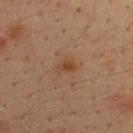No biopsy was performed on this lesion — it was imaged during a full skin examination and was not determined to be concerning. A region of skin cropped from a whole-body photographic capture, roughly 15 mm wide. The tile uses cross-polarized illumination. Automated image analysis of the tile measured about 6 CIELAB-L* units darker than the surrounding skin and a normalized lesion–skin contrast near 7. It also reported border irregularity of about 2 on a 0–10 scale and a color-variation rating of about 3.5/10. The analysis additionally found an automated nevus-likeness rating near 65 out of 100. The patient is a male aged approximately 35. The lesion is on the back.